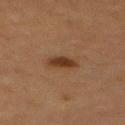Part of a total-body skin-imaging series; this lesion was reviewed on a skin check and was not flagged for biopsy.
Automated image analysis of the tile measured a lesion area of about 5 mm², a shape eccentricity near 0.8, and a shape-asymmetry score of about 0.2 (0 = symmetric). The software also gave an average lesion color of about L≈29 a*≈17 b*≈26 (CIELAB) and a lesion–skin lightness drop of about 9. And it measured a border-irregularity index near 2/10, a within-lesion color-variation index near 2.5/10, and peripheral color asymmetry of about 1. The software also gave an automated nevus-likeness rating near 100 out of 100 and a detector confidence of about 100 out of 100 that the crop contains a lesion.
A female subject about 60 years old.
The lesion's longest dimension is about 3 mm.
A 15 mm close-up tile from a total-body photography series done for melanoma screening.
Located on the upper back.
The tile uses cross-polarized illumination.